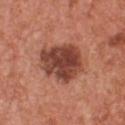A male patient about 55 years old.
The lesion is located on the chest.
A close-up tile cropped from a whole-body skin photograph, about 15 mm across.